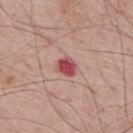Imaged during a routine full-body skin examination; the lesion was not biopsied and no histopathology is available. Located on the mid back. A region of skin cropped from a whole-body photographic capture, roughly 15 mm wide. Approximately 2.5 mm at its widest. A male subject, about 65 years old.